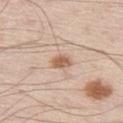<record>
  <biopsy_status>not biopsied; imaged during a skin examination</biopsy_status>
  <lesion_size>
    <long_diameter_mm_approx>2.5</long_diameter_mm_approx>
  </lesion_size>
  <patient>
    <sex>male</sex>
    <age_approx>60</age_approx>
  </patient>
  <site>left thigh</site>
  <lighting>white-light</lighting>
  <image>
    <source>total-body photography crop</source>
    <field_of_view_mm>15</field_of_view_mm>
  </image>
</record>